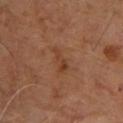Findings:
* follow-up: imaged on a skin check; not biopsied
* tile lighting: cross-polarized
* image: 15 mm crop, total-body photography
* subject: male, in their mid- to late 60s
* diameter: about 3 mm
* image-analysis metrics: a shape-asymmetry score of about 0.55 (0 = symmetric); an average lesion color of about L≈38 a*≈22 b*≈32 (CIELAB), about 7 CIELAB-L* units darker than the surrounding skin, and a normalized lesion–skin contrast near 6.5; border irregularity of about 6.5 on a 0–10 scale, a color-variation rating of about 0/10, and peripheral color asymmetry of about 0; a classifier nevus-likeness of about 0/100 and lesion-presence confidence of about 100/100
* body site: the upper back